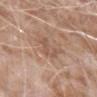notes — imaged on a skin check; not biopsied
site — the left forearm
lighting — white-light
diameter — ≈3 mm
subject — male, in their mid- to late 70s
image source — ~15 mm crop, total-body skin-cancer survey